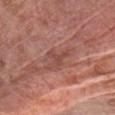{"patient": {"sex": "male", "age_approx": 80}, "image": {"source": "total-body photography crop", "field_of_view_mm": 15}, "lesion_size": {"long_diameter_mm_approx": 2.5}, "site": "chest"}